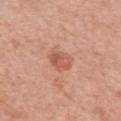| feature | finding |
|---|---|
| anatomic site | the left upper arm |
| lesion size | ≈3 mm |
| patient | female, aged 63 to 67 |
| imaging modality | total-body-photography crop, ~15 mm field of view |